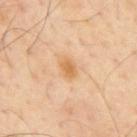biopsy status: imaged on a skin check; not biopsied | anatomic site: the mid back | tile lighting: cross-polarized | subject: male, about 55 years old | automated lesion analysis: a footprint of about 4 mm² and two-axis asymmetry of about 0.2; an average lesion color of about L≈67 a*≈21 b*≈42 (CIELAB), about 9 CIELAB-L* units darker than the surrounding skin, and a lesion-to-skin contrast of about 7 (normalized; higher = more distinct) | image: ~15 mm tile from a whole-body skin photo | lesion diameter: ≈2.5 mm.